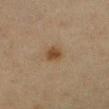This lesion was catalogued during total-body skin photography and was not selected for biopsy.
Imaged with cross-polarized lighting.
The subject is a female approximately 40 years of age.
Cropped from a whole-body photographic skin survey; the tile spans about 15 mm.
The lesion is located on the left lower leg.